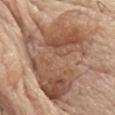Clinical impression: Imaged during a routine full-body skin examination; the lesion was not biopsied and no histopathology is available. Image and clinical context: Automated image analysis of the tile measured a footprint of about 75 mm², an eccentricity of roughly 0.8, and a symmetry-axis asymmetry near 0.35. The software also gave about 12 CIELAB-L* units darker than the surrounding skin and a lesion-to-skin contrast of about 8 (normalized; higher = more distinct). It also reported a border-irregularity rating of about 7.5/10, internal color variation of about 9 on a 0–10 scale, and peripheral color asymmetry of about 3.5. The analysis additionally found a nevus-likeness score of about 10/100 and a detector confidence of about 70 out of 100 that the crop contains a lesion. The lesion is located on the chest. The patient is a male approximately 80 years of age. A region of skin cropped from a whole-body photographic capture, roughly 15 mm wide.